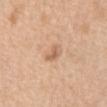<record>
  <biopsy_status>not biopsied; imaged during a skin examination</biopsy_status>
  <lesion_size>
    <long_diameter_mm_approx>2.5</long_diameter_mm_approx>
  </lesion_size>
  <image>
    <source>total-body photography crop</source>
    <field_of_view_mm>15</field_of_view_mm>
  </image>
  <automated_metrics>
    <eccentricity>0.75</eccentricity>
    <shape_asymmetry>0.4</shape_asymmetry>
    <border_irregularity_0_10>3.5</border_irregularity_0_10>
    <color_variation_0_10>3.0</color_variation_0_10>
    <peripheral_color_asymmetry>1.0</peripheral_color_asymmetry>
    <nevus_likeness_0_100>10</nevus_likeness_0_100>
    <lesion_detection_confidence_0_100>100</lesion_detection_confidence_0_100>
  </automated_metrics>
  <site>abdomen</site>
  <lighting>white-light</lighting>
  <patient>
    <sex>female</sex>
    <age_approx>55</age_approx>
  </patient>
</record>